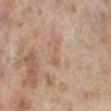{"biopsy_status": "not biopsied; imaged during a skin examination", "site": "leg", "patient": {"sex": "female", "age_approx": 40}, "image": {"source": "total-body photography crop", "field_of_view_mm": 15}, "lighting": "cross-polarized"}